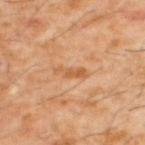Imaged during a routine full-body skin examination; the lesion was not biopsied and no histopathology is available. From the upper back. Imaged with cross-polarized lighting. A 15 mm close-up tile from a total-body photography series done for melanoma screening. The patient is a male aged 58–62. An algorithmic analysis of the crop reported an outline eccentricity of about 0.95 (0 = round, 1 = elongated) and a symmetry-axis asymmetry near 0.35. The recorded lesion diameter is about 3.5 mm.